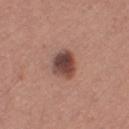  biopsy_status: not biopsied; imaged during a skin examination
  lighting: white-light
  lesion_size:
    long_diameter_mm_approx: 3.0
  automated_metrics:
    cielab_L: 44
    cielab_a: 22
    cielab_b: 23
    vs_skin_darker_L: 15.0
    vs_skin_contrast_norm: 11.5
    border_irregularity_0_10: 1.5
  site: right thigh
  patient:
    sex: female
    age_approx: 30
  image:
    source: total-body photography crop
    field_of_view_mm: 15